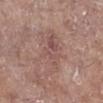Notes:
- follow-up: catalogued during a skin exam; not biopsied
- diameter: ≈6 mm
- tile lighting: white-light illumination
- patient: female, in their mid- to late 70s
- location: the right lower leg
- imaging modality: ~15 mm tile from a whole-body skin photo